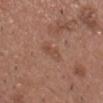Findings:
* notes: no biopsy performed (imaged during a skin exam)
* patient: male, aged 53 to 57
* lesion size: ~3.5 mm (longest diameter)
* anatomic site: the chest
* image-analysis metrics: a lesion area of about 4.5 mm², an outline eccentricity of about 0.9 (0 = round, 1 = elongated), and two-axis asymmetry of about 0.3; an average lesion color of about L≈49 a*≈20 b*≈28 (CIELAB), a lesion–skin lightness drop of about 6, and a normalized border contrast of about 4.5; a classifier nevus-likeness of about 0/100
* lighting: white-light
* imaging modality: 15 mm crop, total-body photography